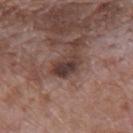No biopsy was performed on this lesion — it was imaged during a full skin examination and was not determined to be concerning. From the mid back. The tile uses white-light illumination. This image is a 15 mm lesion crop taken from a total-body photograph. A male patient about 70 years old.